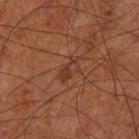{
  "biopsy_status": "not biopsied; imaged during a skin examination",
  "site": "left lower leg",
  "lesion_size": {
    "long_diameter_mm_approx": 3.5
  },
  "image": {
    "source": "total-body photography crop",
    "field_of_view_mm": 15
  },
  "lighting": "cross-polarized",
  "patient": {
    "sex": "male",
    "age_approx": 70
  }
}